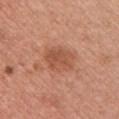Imaged during a routine full-body skin examination; the lesion was not biopsied and no histopathology is available.
The lesion's longest dimension is about 4 mm.
Automated image analysis of the tile measured a shape eccentricity near 0.7 and a shape-asymmetry score of about 0.2 (0 = symmetric).
A female subject, aged approximately 40.
A 15 mm close-up extracted from a 3D total-body photography capture.
The lesion is on the left upper arm.